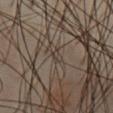Q: Was a biopsy performed?
A: no biopsy performed (imaged during a skin exam)
Q: Lesion location?
A: the abdomen
Q: Patient demographics?
A: male, roughly 45 years of age
Q: What kind of image is this?
A: total-body-photography crop, ~15 mm field of view
Q: How was the tile lit?
A: cross-polarized
Q: How large is the lesion?
A: ~2 mm (longest diameter)